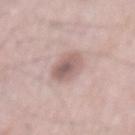subject = male, roughly 65 years of age
body site = the mid back
size = ≈4 mm
tile lighting = white-light illumination
image source = total-body-photography crop, ~15 mm field of view